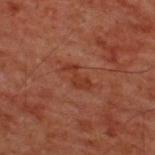| field | value |
|---|---|
| biopsy status | catalogued during a skin exam; not biopsied |
| body site | the back |
| acquisition | total-body-photography crop, ~15 mm field of view |
| subject | male, roughly 60 years of age |